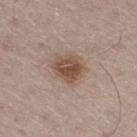Part of a total-body skin-imaging series; this lesion was reviewed on a skin check and was not flagged for biopsy. This image is a 15 mm lesion crop taken from a total-body photograph. A male patient in their mid-60s. Located on the right thigh. The recorded lesion diameter is about 4 mm. This is a white-light tile.